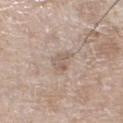Clinical impression:
Imaged during a routine full-body skin examination; the lesion was not biopsied and no histopathology is available.
Clinical summary:
From the leg. A female subject in their mid-70s. Measured at roughly 3 mm in maximum diameter. Imaged with white-light lighting. Cropped from a total-body skin-imaging series; the visible field is about 15 mm.